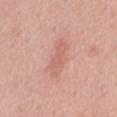biopsy status = no biopsy performed (imaged during a skin exam) | image = 15 mm crop, total-body photography | body site = the back | lighting = white-light | subject = male, approximately 55 years of age.